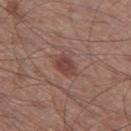Assessment: No biopsy was performed on this lesion — it was imaged during a full skin examination and was not determined to be concerning.